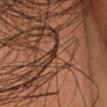The lesion was photographed on a routine skin check and not biopsied; there is no pathology result. A 15 mm close-up tile from a total-body photography series done for melanoma screening. The lesion is on the head or neck. The patient is a male about 40 years old.